A region of skin cropped from a whole-body photographic capture, roughly 15 mm wide · located on the right forearm · the subject is a male aged 63 to 67 — 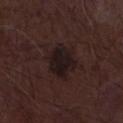{"lighting": "white-light", "automated_metrics": {"area_mm2_approx": 11.0, "eccentricity": 0.6, "cielab_L": 15, "cielab_a": 12, "cielab_b": 11, "vs_skin_darker_L": 7.0, "vs_skin_contrast_norm": 11.0, "nevus_likeness_0_100": 50}, "lesion_size": {"long_diameter_mm_approx": 4.5}, "diagnosis": {"histopathology": "seborrheic keratosis", "malignancy": "benign", "taxonomic_path": ["Benign", "Benign epidermal proliferations", "Seborrheic keratosis"]}}